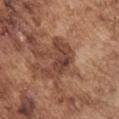| key | value |
|---|---|
| location | the left upper arm |
| subject | male, aged 73–77 |
| image | 15 mm crop, total-body photography |